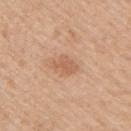No biopsy was performed on this lesion — it was imaged during a full skin examination and was not determined to be concerning.
The lesion is on the right upper arm.
A 15 mm crop from a total-body photograph taken for skin-cancer surveillance.
A female subject, in their 50s.